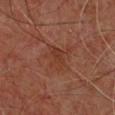Part of a total-body skin-imaging series; this lesion was reviewed on a skin check and was not flagged for biopsy.
Imaged with cross-polarized lighting.
Cropped from a whole-body photographic skin survey; the tile spans about 15 mm.
Automated tile analysis of the lesion measured a border-irregularity index near 2.5/10 and internal color variation of about 2.5 on a 0–10 scale. And it measured a classifier nevus-likeness of about 0/100 and lesion-presence confidence of about 100/100.
The lesion is located on the back.
A male patient, in their 60s.
Approximately 3.5 mm at its widest.